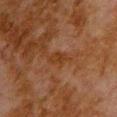biopsy status = total-body-photography surveillance lesion; no biopsy | lighting = cross-polarized illumination | location = the back | acquisition = 15 mm crop, total-body photography | automated metrics = border irregularity of about 2.5 on a 0–10 scale and internal color variation of about 2 on a 0–10 scale; a classifier nevus-likeness of about 0/100 | subject = male, approximately 80 years of age | size = ≈2.5 mm.